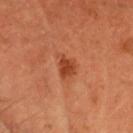Assessment: This lesion was catalogued during total-body skin photography and was not selected for biopsy. Context: A male subject, in their mid- to late 50s. Measured at roughly 2.5 mm in maximum diameter. A 15 mm crop from a total-body photograph taken for skin-cancer surveillance. The lesion is on the head or neck.